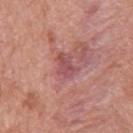{"patient": {"sex": "male", "age_approx": 65}, "image": {"source": "total-body photography crop", "field_of_view_mm": 15}, "site": "mid back"}